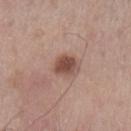| key | value |
|---|---|
| follow-up | no biopsy performed (imaged during a skin exam) |
| imaging modality | ~15 mm crop, total-body skin-cancer survey |
| subject | male, approximately 60 years of age |
| lesion diameter | about 3 mm |
| body site | the left lower leg |
| image-analysis metrics | an automated nevus-likeness rating near 95 out of 100 and a lesion-detection confidence of about 100/100 |
| tile lighting | white-light illumination |